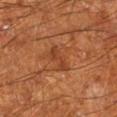Case summary:
- follow-up: catalogued during a skin exam; not biopsied
- image-analysis metrics: a lesion-to-skin contrast of about 6 (normalized; higher = more distinct)
- site: the left lower leg
- imaging modality: ~15 mm tile from a whole-body skin photo
- lesion size: about 3.5 mm
- subject: male, aged 63–67
- lighting: cross-polarized